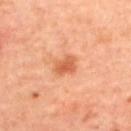This lesion was catalogued during total-body skin photography and was not selected for biopsy.